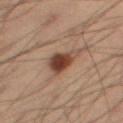The lesion was tiled from a total-body skin photograph and was not biopsied.
Captured under cross-polarized illumination.
A region of skin cropped from a whole-body photographic capture, roughly 15 mm wide.
The recorded lesion diameter is about 3.5 mm.
From the right thigh.
The patient is a male about 55 years old.